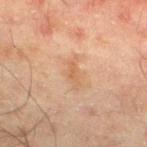acquisition: 15 mm crop, total-body photography | subject: male, aged approximately 45 | location: the left lower leg.